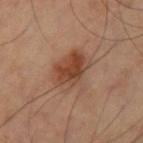notes=total-body-photography surveillance lesion; no biopsy
anatomic site=the right thigh
tile lighting=cross-polarized
imaging modality=total-body-photography crop, ~15 mm field of view
diameter=~5 mm (longest diameter)
automated metrics=an area of roughly 14 mm², an eccentricity of roughly 0.65, and two-axis asymmetry of about 0.25; a border-irregularity rating of about 3.5/10, internal color variation of about 6 on a 0–10 scale, and a peripheral color-asymmetry measure near 2; a detector confidence of about 100 out of 100 that the crop contains a lesion